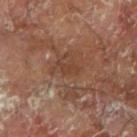<case>
  <biopsy_status>not biopsied; imaged during a skin examination</biopsy_status>
  <automated_metrics>
    <border_irregularity_0_10>4.0</border_irregularity_0_10>
    <color_variation_0_10>1.0</color_variation_0_10>
    <peripheral_color_asymmetry>0.5</peripheral_color_asymmetry>
  </automated_metrics>
  <lesion_size>
    <long_diameter_mm_approx>2.5</long_diameter_mm_approx>
  </lesion_size>
  <image>
    <source>total-body photography crop</source>
    <field_of_view_mm>15</field_of_view_mm>
  </image>
  <patient>
    <sex>male</sex>
    <age_approx>65</age_approx>
  </patient>
  <site>right forearm</site>
  <lighting>cross-polarized</lighting>
</case>